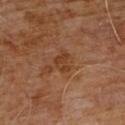Impression:
Captured during whole-body skin photography for melanoma surveillance; the lesion was not biopsied.
Clinical summary:
A region of skin cropped from a whole-body photographic capture, roughly 15 mm wide. Measured at roughly 2.5 mm in maximum diameter. The subject is a male about 60 years old. The lesion is on the chest.